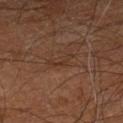diameter: ≈3 mm
site: the right leg
image-analysis metrics: an automated nevus-likeness rating near 0 out of 100
subject: male, roughly 60 years of age
lighting: cross-polarized illumination
acquisition: total-body-photography crop, ~15 mm field of view